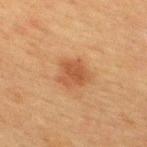A 15 mm close-up tile from a total-body photography series done for melanoma screening.
Located on the upper back.
Approximately 3.5 mm at its widest.
Imaged with cross-polarized lighting.
A female patient, in their mid-50s.